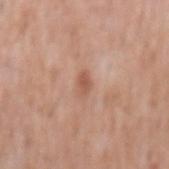This lesion was catalogued during total-body skin photography and was not selected for biopsy. Longest diameter approximately 2.5 mm. A 15 mm crop from a total-body photograph taken for skin-cancer surveillance. The total-body-photography lesion software estimated an area of roughly 3 mm², a shape eccentricity near 0.85, and two-axis asymmetry of about 0.3. And it measured a mean CIELAB color near L≈56 a*≈23 b*≈30, a lesion–skin lightness drop of about 9, and a normalized border contrast of about 6.5. And it measured a nevus-likeness score of about 0/100 and lesion-presence confidence of about 100/100. A male patient aged around 55. The lesion is on the mid back.